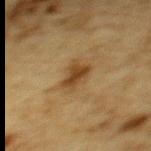Imaged during a routine full-body skin examination; the lesion was not biopsied and no histopathology is available.
This image is a 15 mm lesion crop taken from a total-body photograph.
A male patient about 85 years old or older.
From the upper back.